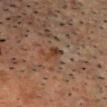The lesion was tiled from a total-body skin photograph and was not biopsied. On the head or neck. A male subject, aged 53 to 57. Longest diameter approximately 3 mm. The tile uses cross-polarized illumination. A close-up tile cropped from a whole-body skin photograph, about 15 mm across.